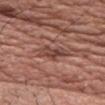Q: Was this lesion biopsied?
A: imaged on a skin check; not biopsied
Q: How was this image acquired?
A: 15 mm crop, total-body photography
Q: Illumination type?
A: white-light
Q: Patient demographics?
A: male, approximately 70 years of age
Q: Where on the body is the lesion?
A: the chest
Q: What is the lesion's diameter?
A: ≈4.5 mm
Q: What did automated image analysis measure?
A: a mean CIELAB color near L≈44 a*≈22 b*≈25 and a normalized border contrast of about 7; an automated nevus-likeness rating near 0 out of 100 and lesion-presence confidence of about 70/100